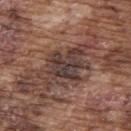Impression:
This lesion was catalogued during total-body skin photography and was not selected for biopsy.
Image and clinical context:
A lesion tile, about 15 mm wide, cut from a 3D total-body photograph. The lesion is located on the upper back. A male subject about 75 years old.